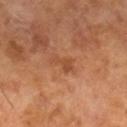Notes:
• workup: total-body-photography surveillance lesion; no biopsy
• size: about 3 mm
• image: total-body-photography crop, ~15 mm field of view
• lighting: cross-polarized illumination
• TBP lesion metrics: a mean CIELAB color near L≈47 a*≈24 b*≈35, about 8 CIELAB-L* units darker than the surrounding skin, and a normalized border contrast of about 6; a border-irregularity rating of about 5.5/10, internal color variation of about 0.5 on a 0–10 scale, and a peripheral color-asymmetry measure near 0
• subject: male, aged 63–67